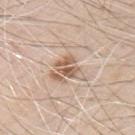This lesion was catalogued during total-body skin photography and was not selected for biopsy. The total-body-photography lesion software estimated an eccentricity of roughly 0.45. The analysis additionally found about 12 CIELAB-L* units darker than the surrounding skin and a normalized border contrast of about 8. The software also gave lesion-presence confidence of about 75/100. From the left upper arm. The patient is a male aged around 70. About 2.5 mm across. Captured under white-light illumination. A 15 mm close-up extracted from a 3D total-body photography capture.